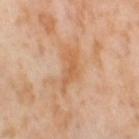Captured during whole-body skin photography for melanoma surveillance; the lesion was not biopsied. The lesion is on the right thigh. A 15 mm close-up tile from a total-body photography series done for melanoma screening. This is a cross-polarized tile. The patient is a female about 55 years old. Longest diameter approximately 5 mm.